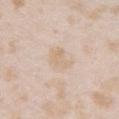The lesion was tiled from a total-body skin photograph and was not biopsied. Cropped from a whole-body photographic skin survey; the tile spans about 15 mm. The lesion is on the chest. An algorithmic analysis of the crop reported a footprint of about 6 mm², an outline eccentricity of about 0.65 (0 = round, 1 = elongated), and a symmetry-axis asymmetry near 0.35. The software also gave an average lesion color of about L≈71 a*≈13 b*≈30 (CIELAB), roughly 6 lightness units darker than nearby skin, and a lesion-to-skin contrast of about 4.5 (normalized; higher = more distinct). The subject is a female aged 23–27. The tile uses white-light illumination. About 3 mm across.